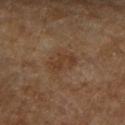Case summary:
– notes — no biopsy performed (imaged during a skin exam)
– image — ~15 mm tile from a whole-body skin photo
– site — the right forearm
– subject — male, roughly 85 years of age or older